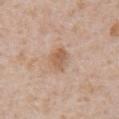notes: catalogued during a skin exam; not biopsied | lesion diameter: ≈3 mm | image source: ~15 mm tile from a whole-body skin photo | lighting: white-light illumination | site: the chest | subject: male, aged approximately 65 | automated metrics: a border-irregularity index near 2/10, a color-variation rating of about 3.5/10, and a peripheral color-asymmetry measure near 1; a detector confidence of about 100 out of 100 that the crop contains a lesion.